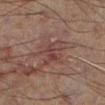<tbp_lesion>
<biopsy_status>not biopsied; imaged during a skin examination</biopsy_status>
<lesion_size>
  <long_diameter_mm_approx>5.0</long_diameter_mm_approx>
</lesion_size>
<patient>
  <sex>male</sex>
  <age_approx>60</age_approx>
</patient>
<automated_metrics>
  <area_mm2_approx>9.0</area_mm2_approx>
  <eccentricity>0.8</eccentricity>
  <shape_asymmetry>0.3</shape_asymmetry>
  <cielab_L>30</cielab_L>
  <cielab_a>15</cielab_a>
  <cielab_b>17</cielab_b>
  <vs_skin_darker_L>5.0</vs_skin_darker_L>
</automated_metrics>
<image>
  <source>total-body photography crop</source>
  <field_of_view_mm>15</field_of_view_mm>
</image>
<site>left lower leg</site>
<lighting>cross-polarized</lighting>
</tbp_lesion>